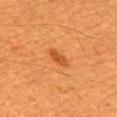Clinical impression:
Imaged during a routine full-body skin examination; the lesion was not biopsied and no histopathology is available.
Clinical summary:
The lesion is on the upper back. Captured under cross-polarized illumination. Measured at roughly 3 mm in maximum diameter. A 15 mm close-up extracted from a 3D total-body photography capture. The total-body-photography lesion software estimated an outline eccentricity of about 0.85 (0 = round, 1 = elongated). The software also gave border irregularity of about 1.5 on a 0–10 scale, a color-variation rating of about 1.5/10, and peripheral color asymmetry of about 0.5. It also reported a nevus-likeness score of about 95/100 and a detector confidence of about 100 out of 100 that the crop contains a lesion. A male subject, about 60 years old.